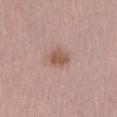notes: catalogued during a skin exam; not biopsied | location: the abdomen | TBP lesion metrics: an average lesion color of about L≈55 a*≈21 b*≈27 (CIELAB), about 10 CIELAB-L* units darker than the surrounding skin, and a normalized lesion–skin contrast near 7.5; a lesion-detection confidence of about 100/100 | subject: female, aged 38 to 42 | acquisition: 15 mm crop, total-body photography.